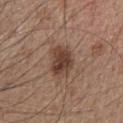workup: catalogued during a skin exam; not biopsied
diameter: ≈4 mm
site: the head or neck
tile lighting: white-light illumination
image source: 15 mm crop, total-body photography
automated metrics: a lesion area of about 10 mm², a shape eccentricity near 0.7, and a shape-asymmetry score of about 0.15 (0 = symmetric); a mean CIELAB color near L≈42 a*≈18 b*≈25, roughly 12 lightness units darker than nearby skin, and a normalized border contrast of about 9; a border-irregularity rating of about 2/10, a color-variation rating of about 4.5/10, and a peripheral color-asymmetry measure near 1.5
patient: female, approximately 40 years of age